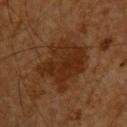Findings:
* follow-up — catalogued during a skin exam; not biopsied
* image — total-body-photography crop, ~15 mm field of view
* tile lighting — cross-polarized illumination
* anatomic site — the head or neck
* subject — male, in their mid- to late 60s
* lesion diameter — ≈6.5 mm